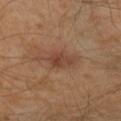{"biopsy_status": "not biopsied; imaged during a skin examination", "site": "right lower leg", "lighting": "cross-polarized", "image": {"source": "total-body photography crop", "field_of_view_mm": 15}, "patient": {"sex": "male", "age_approx": 55}}